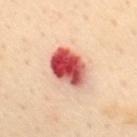Impression:
The lesion was tiled from a total-body skin photograph and was not biopsied.
Image and clinical context:
The lesion's longest dimension is about 5 mm. A close-up tile cropped from a whole-body skin photograph, about 15 mm across. On the mid back. This is a cross-polarized tile. A female subject, about 60 years old.